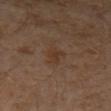Imaged during a routine full-body skin examination; the lesion was not biopsied and no histopathology is available.
A lesion tile, about 15 mm wide, cut from a 3D total-body photograph.
A male subject, roughly 30 years of age.
On the leg.